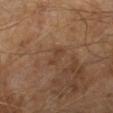Q: Was this lesion biopsied?
A: no biopsy performed (imaged during a skin exam)
Q: What is the lesion's diameter?
A: ~2.5 mm (longest diameter)
Q: What kind of image is this?
A: 15 mm crop, total-body photography
Q: Illumination type?
A: cross-polarized
Q: Who is the patient?
A: male, in their mid-60s